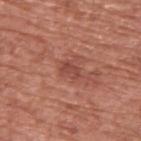Cropped from a total-body skin-imaging series; the visible field is about 15 mm.
The patient is a male about 75 years old.
From the upper back.